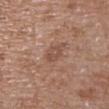{"biopsy_status": "not biopsied; imaged during a skin examination", "image": {"source": "total-body photography crop", "field_of_view_mm": 15}, "patient": {"sex": "female", "age_approx": 65}, "site": "upper back", "lighting": "white-light"}